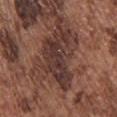A lesion tile, about 15 mm wide, cut from a 3D total-body photograph.
A male patient approximately 75 years of age.
Imaged with white-light lighting.
Located on the upper back.